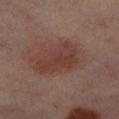Q: Was this lesion biopsied?
A: imaged on a skin check; not biopsied
Q: What kind of image is this?
A: 15 mm crop, total-body photography
Q: Where on the body is the lesion?
A: the left lower leg
Q: Lesion size?
A: about 5 mm
Q: Patient demographics?
A: female, aged around 50
Q: What lighting was used for the tile?
A: cross-polarized
Q: What did automated image analysis measure?
A: an area of roughly 14 mm², an outline eccentricity of about 0.7 (0 = round, 1 = elongated), and two-axis asymmetry of about 0.35; an automated nevus-likeness rating near 30 out of 100